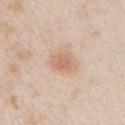{"biopsy_status": "not biopsied; imaged during a skin examination", "patient": {"sex": "male", "age_approx": 25}, "image": {"source": "total-body photography crop", "field_of_view_mm": 15}, "site": "chest"}